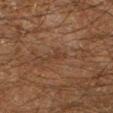workup: imaged on a skin check; not biopsied | imaging modality: ~15 mm tile from a whole-body skin photo | location: the left lower leg | patient: male, about 60 years old | lesion size: ≈3 mm.